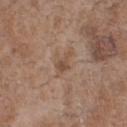| feature | finding |
|---|---|
| biopsy status | total-body-photography surveillance lesion; no biopsy |